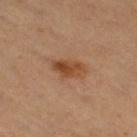Part of a total-body skin-imaging series; this lesion was reviewed on a skin check and was not flagged for biopsy. The patient is a female about 50 years old. A roughly 15 mm field-of-view crop from a total-body skin photograph. The lesion-visualizer software estimated a shape eccentricity near 0.85. The analysis additionally found a border-irregularity rating of about 2/10 and peripheral color asymmetry of about 2. The recorded lesion diameter is about 3.5 mm. Imaged with cross-polarized lighting. The lesion is on the right thigh.